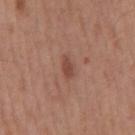Acquisition and patient details: A 15 mm crop from a total-body photograph taken for skin-cancer surveillance. The subject is a male approximately 60 years of age. The lesion is located on the mid back.